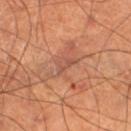  biopsy_status: not biopsied; imaged during a skin examination
  site: right thigh
  patient:
    sex: male
    age_approx: 70
  image:
    source: total-body photography crop
    field_of_view_mm: 15